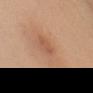{
  "biopsy_status": "not biopsied; imaged during a skin examination",
  "automated_metrics": {
    "eccentricity": 0.9,
    "shape_asymmetry": 0.25,
    "cielab_L": 55,
    "cielab_a": 23,
    "cielab_b": 33,
    "vs_skin_darker_L": 7.0,
    "vs_skin_contrast_norm": 5.0,
    "border_irregularity_0_10": 3.0,
    "color_variation_0_10": 0.0,
    "nevus_likeness_0_100": 15,
    "lesion_detection_confidence_0_100": 100
  },
  "site": "chest",
  "image": {
    "source": "total-body photography crop",
    "field_of_view_mm": 15
  },
  "lesion_size": {
    "long_diameter_mm_approx": 3.0
  },
  "patient": {
    "sex": "female",
    "age_approx": 45
  }
}